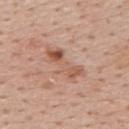  patient:
    sex: male
    age_approx: 55
  site: upper back
  image:
    source: total-body photography crop
    field_of_view_mm: 15